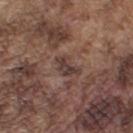Captured during whole-body skin photography for melanoma surveillance; the lesion was not biopsied.
Automated tile analysis of the lesion measured a symmetry-axis asymmetry near 0.45. It also reported a mean CIELAB color near L≈38 a*≈16 b*≈20, a lesion–skin lightness drop of about 9, and a lesion-to-skin contrast of about 8 (normalized; higher = more distinct). And it measured a within-lesion color-variation index near 3.5/10 and radial color variation of about 1. It also reported a nevus-likeness score of about 0/100.
Measured at roughly 3.5 mm in maximum diameter.
This is a white-light tile.
A 15 mm close-up extracted from a 3D total-body photography capture.
The lesion is on the arm.
A male subject, aged 73–77.